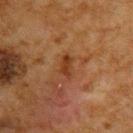notes = no biopsy performed (imaged during a skin exam)
patient = male, roughly 60 years of age
tile lighting = cross-polarized
image-analysis metrics = an average lesion color of about L≈32 a*≈21 b*≈31 (CIELAB), roughly 7 lightness units darker than nearby skin, and a normalized lesion–skin contrast near 7.5
anatomic site = the back
diameter = about 2.5 mm
acquisition = total-body-photography crop, ~15 mm field of view